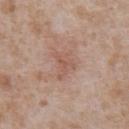follow-up: catalogued during a skin exam; not biopsied.